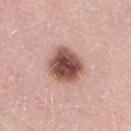workup: imaged on a skin check; not biopsied | body site: the leg | patient: male, aged approximately 50 | automated lesion analysis: a footprint of about 14 mm² and a shape eccentricity near 0.35; an average lesion color of about L≈51 a*≈23 b*≈25 (CIELAB), about 19 CIELAB-L* units darker than the surrounding skin, and a normalized border contrast of about 12 | imaging modality: 15 mm crop, total-body photography | lesion size: ~4 mm (longest diameter) | illumination: white-light.